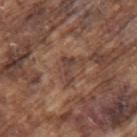Q: Was a biopsy performed?
A: imaged on a skin check; not biopsied
Q: Lesion size?
A: ~3 mm (longest diameter)
Q: What kind of image is this?
A: total-body-photography crop, ~15 mm field of view
Q: Patient demographics?
A: male, in their mid-70s
Q: Illumination type?
A: white-light illumination
Q: Where on the body is the lesion?
A: the left upper arm